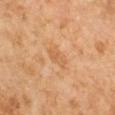Assessment:
This lesion was catalogued during total-body skin photography and was not selected for biopsy.
Background:
An algorithmic analysis of the crop reported an average lesion color of about L≈58 a*≈21 b*≈38 (CIELAB), roughly 6 lightness units darker than nearby skin, and a normalized lesion–skin contrast near 4.5. The analysis additionally found an automated nevus-likeness rating near 0 out of 100 and lesion-presence confidence of about 100/100. The tile uses cross-polarized illumination. A 15 mm close-up extracted from a 3D total-body photography capture. On the right arm. The recorded lesion diameter is about 3 mm. A male patient, aged 58–62.